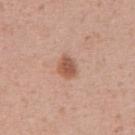<case>
  <biopsy_status>not biopsied; imaged during a skin examination</biopsy_status>
  <image>
    <source>total-body photography crop</source>
    <field_of_view_mm>15</field_of_view_mm>
  </image>
  <lesion_size>
    <long_diameter_mm_approx>3.0</long_diameter_mm_approx>
  </lesion_size>
  <site>abdomen</site>
  <patient>
    <sex>male</sex>
    <age_approx>55</age_approx>
  </patient>
</case>